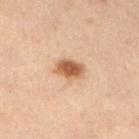{"biopsy_status": "not biopsied; imaged during a skin examination", "patient": {"sex": "male", "age_approx": 55}, "image": {"source": "total-body photography crop", "field_of_view_mm": 15}, "site": "left lower leg"}